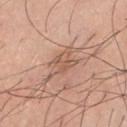Recorded during total-body skin imaging; not selected for excision or biopsy.
A male subject, aged 43 to 47.
The total-body-photography lesion software estimated a mean CIELAB color near L≈57 a*≈20 b*≈29, a lesion–skin lightness drop of about 9, and a normalized lesion–skin contrast near 6. The analysis additionally found a border-irregularity index near 6.5/10, a color-variation rating of about 2.5/10, and radial color variation of about 1.
Imaged with white-light lighting.
A roughly 15 mm field-of-view crop from a total-body skin photograph.
About 4.5 mm across.
Located on the chest.